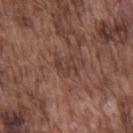biopsy_status: not biopsied; imaged during a skin examination
site: chest
lesion_size:
  long_diameter_mm_approx: 2.5
lighting: white-light
image:
  source: total-body photography crop
  field_of_view_mm: 15
automated_metrics:
  cielab_L: 39
  cielab_a: 19
  cielab_b: 23
  vs_skin_darker_L: 6.0
  vs_skin_contrast_norm: 6.0
  nevus_likeness_0_100: 0
  lesion_detection_confidence_0_100: 85
patient:
  sex: male
  age_approx: 75